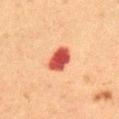No biopsy was performed on this lesion — it was imaged during a full skin examination and was not determined to be concerning.
A female subject aged 48–52.
From the abdomen.
Cropped from a total-body skin-imaging series; the visible field is about 15 mm.
The total-body-photography lesion software estimated about 18 CIELAB-L* units darker than the surrounding skin and a lesion-to-skin contrast of about 12.5 (normalized; higher = more distinct). The analysis additionally found a border-irregularity index near 2.5/10, a color-variation rating of about 3/10, and radial color variation of about 1. It also reported a detector confidence of about 100 out of 100 that the crop contains a lesion.